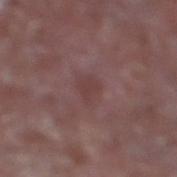Acquisition and patient details: The lesion is located on the right lower leg. Longest diameter approximately 3 mm. A male subject, aged 63–67. Imaged with white-light lighting. The lesion-visualizer software estimated an area of roughly 4.5 mm², an outline eccentricity of about 0.6 (0 = round, 1 = elongated), and a shape-asymmetry score of about 0.35 (0 = symmetric). The analysis additionally found a border-irregularity rating of about 3/10, a within-lesion color-variation index near 1.5/10, and radial color variation of about 0.5. It also reported a classifier nevus-likeness of about 0/100 and lesion-presence confidence of about 100/100. A roughly 15 mm field-of-view crop from a total-body skin photograph.